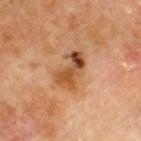Q: Was a biopsy performed?
A: no biopsy performed (imaged during a skin exam)
Q: Lesion location?
A: the upper back
Q: Patient demographics?
A: male, roughly 70 years of age
Q: How was this image acquired?
A: 15 mm crop, total-body photography